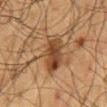Assessment: This lesion was catalogued during total-body skin photography and was not selected for biopsy. Background: A male patient aged approximately 60. The tile uses cross-polarized illumination. Located on the mid back. Longest diameter approximately 5 mm. This image is a 15 mm lesion crop taken from a total-body photograph.